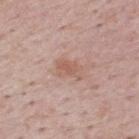This lesion was catalogued during total-body skin photography and was not selected for biopsy. Measured at roughly 3 mm in maximum diameter. The lesion is on the upper back. Imaged with white-light lighting. A female patient approximately 50 years of age. A lesion tile, about 15 mm wide, cut from a 3D total-body photograph. Automated image analysis of the tile measured a nevus-likeness score of about 5/100 and a lesion-detection confidence of about 100/100.